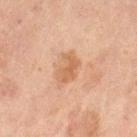Findings:
– workup — total-body-photography surveillance lesion; no biopsy
– lesion diameter — ~3.5 mm (longest diameter)
– image — ~15 mm crop, total-body skin-cancer survey
– lighting — cross-polarized illumination
– location — the left thigh
– TBP lesion metrics — a footprint of about 5.5 mm² and a symmetry-axis asymmetry near 0.35; an average lesion color of about L≈54 a*≈20 b*≈33 (CIELAB), a lesion–skin lightness drop of about 8, and a lesion-to-skin contrast of about 6.5 (normalized; higher = more distinct); a border-irregularity index near 3.5/10
– subject — female, aged approximately 60